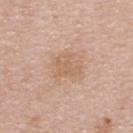Notes:
* acquisition: ~15 mm crop, total-body skin-cancer survey
* body site: the upper back
* patient: female, aged approximately 45
* tile lighting: white-light illumination
* lesion size: ~3 mm (longest diameter)